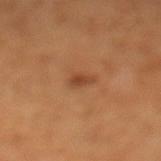Captured during whole-body skin photography for melanoma surveillance; the lesion was not biopsied. A 15 mm crop from a total-body photograph taken for skin-cancer surveillance. Automated tile analysis of the lesion measured a footprint of about 2.5 mm², an eccentricity of roughly 0.7, and a symmetry-axis asymmetry near 0.3. It also reported an average lesion color of about L≈43 a*≈24 b*≈35 (CIELAB), roughly 9 lightness units darker than nearby skin, and a normalized border contrast of about 7. And it measured a border-irregularity index near 2.5/10, a within-lesion color-variation index near 1/10, and a peripheral color-asymmetry measure near 0.5. The lesion is on the leg. A female subject, aged 63–67. About 2 mm across.